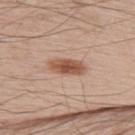Part of a total-body skin-imaging series; this lesion was reviewed on a skin check and was not flagged for biopsy. The patient is a male aged 63–67. Cropped from a total-body skin-imaging series; the visible field is about 15 mm. Captured under white-light illumination. The lesion is located on the back. Longest diameter approximately 4 mm. The lesion-visualizer software estimated a lesion area of about 7.5 mm², an eccentricity of roughly 0.9, and a shape-asymmetry score of about 0.2 (0 = symmetric). The analysis additionally found a lesion color around L≈54 a*≈21 b*≈30 in CIELAB, about 13 CIELAB-L* units darker than the surrounding skin, and a normalized border contrast of about 9. And it measured a border-irregularity index near 2/10 and internal color variation of about 4.5 on a 0–10 scale.